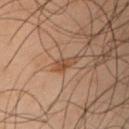Impression:
Recorded during total-body skin imaging; not selected for excision or biopsy.
Context:
Measured at roughly 2.5 mm in maximum diameter. Imaged with cross-polarized lighting. An algorithmic analysis of the crop reported a border-irregularity rating of about 3/10 and a color-variation rating of about 1/10. And it measured a nevus-likeness score of about 70/100 and a detector confidence of about 95 out of 100 that the crop contains a lesion. A 15 mm close-up extracted from a 3D total-body photography capture. Located on the left forearm. The subject is a male in their 50s.